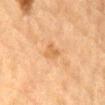Imaged during a routine full-body skin examination; the lesion was not biopsied and no histopathology is available. The subject is a male aged approximately 85 or older. A 15 mm close-up extracted from a 3D total-body photography capture. Captured under cross-polarized illumination. From the mid back. Measured at roughly 2.5 mm in maximum diameter.